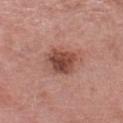biopsy status = catalogued during a skin exam; not biopsied | automated lesion analysis = an automated nevus-likeness rating near 75 out of 100 | diameter = ~4.5 mm (longest diameter) | lighting = white-light illumination | imaging modality = ~15 mm tile from a whole-body skin photo | body site = the left thigh | subject = female, aged 68–72.